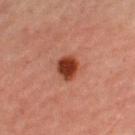Q: Was this lesion biopsied?
A: catalogued during a skin exam; not biopsied
Q: Lesion location?
A: the left thigh
Q: Patient demographics?
A: female, approximately 70 years of age
Q: What did automated image analysis measure?
A: about 15 CIELAB-L* units darker than the surrounding skin; a color-variation rating of about 3/10 and peripheral color asymmetry of about 1
Q: What is the imaging modality?
A: total-body-photography crop, ~15 mm field of view
Q: What is the lesion's diameter?
A: about 3 mm
Q: What lighting was used for the tile?
A: cross-polarized